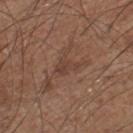| feature | finding |
|---|---|
| workup | no biopsy performed (imaged during a skin exam) |
| anatomic site | the right lower leg |
| patient | male, aged 58–62 |
| lesion size | ≈2.5 mm |
| image-analysis metrics | an average lesion color of about L≈41 a*≈19 b*≈25 (CIELAB), a lesion–skin lightness drop of about 6, and a normalized lesion–skin contrast near 5.5; border irregularity of about 5 on a 0–10 scale, internal color variation of about 1.5 on a 0–10 scale, and peripheral color asymmetry of about 0.5; a nevus-likeness score of about 0/100 and a lesion-detection confidence of about 90/100 |
| imaging modality | 15 mm crop, total-body photography |
| illumination | white-light |